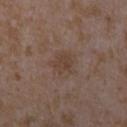Q: Was a biopsy performed?
A: no biopsy performed (imaged during a skin exam)
Q: What kind of image is this?
A: total-body-photography crop, ~15 mm field of view
Q: Automated lesion metrics?
A: two-axis asymmetry of about 0.3; border irregularity of about 2.5 on a 0–10 scale, internal color variation of about 2 on a 0–10 scale, and a peripheral color-asymmetry measure near 0.5; a nevus-likeness score of about 0/100
Q: What is the anatomic site?
A: the right forearm
Q: Patient demographics?
A: female, aged approximately 35
Q: How was the tile lit?
A: white-light
Q: Lesion size?
A: ≈2.5 mm